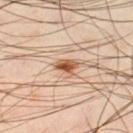  biopsy_status: not biopsied; imaged during a skin examination
  patient:
    sex: male
    age_approx: 50
  lighting: cross-polarized
  lesion_size:
    long_diameter_mm_approx: 2.5
  image:
    source: total-body photography crop
    field_of_view_mm: 15
  site: left thigh